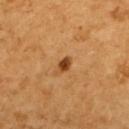Part of a total-body skin-imaging series; this lesion was reviewed on a skin check and was not flagged for biopsy. A female patient, about 55 years old. The lesion is located on the upper back. Automated image analysis of the tile measured a lesion color around L≈46 a*≈26 b*≈42 in CIELAB, about 14 CIELAB-L* units darker than the surrounding skin, and a lesion-to-skin contrast of about 10 (normalized; higher = more distinct). The software also gave border irregularity of about 2.5 on a 0–10 scale, a color-variation rating of about 1.5/10, and peripheral color asymmetry of about 0.5. The analysis additionally found an automated nevus-likeness rating near 95 out of 100. A lesion tile, about 15 mm wide, cut from a 3D total-body photograph. About 2 mm across.